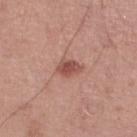Impression:
Part of a total-body skin-imaging series; this lesion was reviewed on a skin check and was not flagged for biopsy.
Context:
The recorded lesion diameter is about 3 mm. A male subject, about 50 years old. From the right lower leg. The tile uses white-light illumination. Automated image analysis of the tile measured a lesion area of about 5 mm², an outline eccentricity of about 0.7 (0 = round, 1 = elongated), and two-axis asymmetry of about 0.15. A 15 mm close-up tile from a total-body photography series done for melanoma screening.